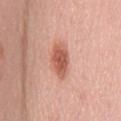biopsy_status: not biopsied; imaged during a skin examination
lesion_size:
  long_diameter_mm_approx: 5.0
site: back
patient:
  sex: female
  age_approx: 50
image:
  source: total-body photography crop
  field_of_view_mm: 15
automated_metrics:
  area_mm2_approx: 8.5
  eccentricity: 0.9
  shape_asymmetry: 0.25
  border_irregularity_0_10: 3.0
  color_variation_0_10: 3.5
  peripheral_color_asymmetry: 1.0
  nevus_likeness_0_100: 90
  lesion_detection_confidence_0_100: 100
lighting: white-light